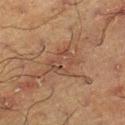Case summary:
– imaging modality: ~15 mm tile from a whole-body skin photo
– location: the right lower leg
– subject: male, approximately 60 years of age
– lighting: cross-polarized
– image-analysis metrics: a footprint of about 8 mm² and two-axis asymmetry of about 0.3; roughly 5 lightness units darker than nearby skin and a lesion-to-skin contrast of about 4.5 (normalized; higher = more distinct); a border-irregularity index near 3/10, a within-lesion color-variation index near 3.5/10, and a peripheral color-asymmetry measure near 1.5; an automated nevus-likeness rating near 0 out of 100 and a detector confidence of about 55 out of 100 that the crop contains a lesion